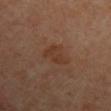patient — male, aged approximately 65
diameter — ≈3.5 mm
body site — the left upper arm
tile lighting — cross-polarized
image-analysis metrics — a mean CIELAB color near L≈35 a*≈18 b*≈27, about 5 CIELAB-L* units darker than the surrounding skin, and a normalized border contrast of about 6; a border-irregularity rating of about 4.5/10, a within-lesion color-variation index near 2/10, and a peripheral color-asymmetry measure near 1
image source — ~15 mm crop, total-body skin-cancer survey